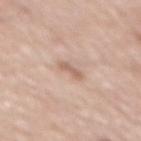notes: catalogued during a skin exam; not biopsied
image: ~15 mm tile from a whole-body skin photo
diameter: ~3 mm (longest diameter)
subject: male, about 70 years old
location: the mid back
tile lighting: white-light illumination